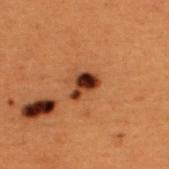Notes:
• biopsy status: no biopsy performed (imaged during a skin exam)
• image-analysis metrics: a lesion–skin lightness drop of about 17 and a lesion-to-skin contrast of about 16 (normalized; higher = more distinct); a within-lesion color-variation index near 4/10 and a peripheral color-asymmetry measure near 1; an automated nevus-likeness rating near 80 out of 100 and a detector confidence of about 100 out of 100 that the crop contains a lesion
• image source: 15 mm crop, total-body photography
• site: the upper back
• subject: male, aged approximately 50